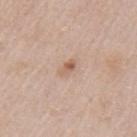{"biopsy_status": "not biopsied; imaged during a skin examination", "lesion_size": {"long_diameter_mm_approx": 2.5}, "patient": {"sex": "female", "age_approx": 40}, "site": "left upper arm", "lighting": "white-light", "image": {"source": "total-body photography crop", "field_of_view_mm": 15}}